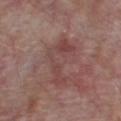Captured during whole-body skin photography for melanoma surveillance; the lesion was not biopsied.
A lesion tile, about 15 mm wide, cut from a 3D total-body photograph.
A male subject approximately 65 years of age.
Approximately 6 mm at its widest.
On the chest.